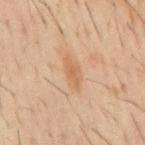Imaged with cross-polarized lighting. An algorithmic analysis of the crop reported a border-irregularity rating of about 2.5/10, a within-lesion color-variation index near 2/10, and radial color variation of about 0.5. Approximately 4 mm at its widest. A male patient, about 60 years old. The lesion is located on the mid back. A lesion tile, about 15 mm wide, cut from a 3D total-body photograph.